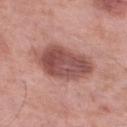{"biopsy_status": "not biopsied; imaged during a skin examination", "patient": {"sex": "male", "age_approx": 55}, "site": "right lower leg", "lesion_size": {"long_diameter_mm_approx": 6.5}, "image": {"source": "total-body photography crop", "field_of_view_mm": 15}}